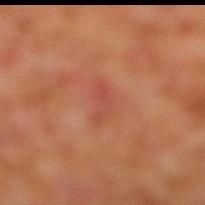This lesion was catalogued during total-body skin photography and was not selected for biopsy. Automated tile analysis of the lesion measured a border-irregularity index near 5.5/10 and peripheral color asymmetry of about 0.5. It also reported a nevus-likeness score of about 0/100 and a detector confidence of about 100 out of 100 that the crop contains a lesion. A roughly 15 mm field-of-view crop from a total-body skin photograph. The subject is a male about 60 years old. Captured under cross-polarized illumination. The lesion is on the right lower leg. Approximately 3.5 mm at its widest.